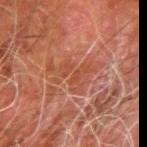Clinical impression: Captured during whole-body skin photography for melanoma surveillance; the lesion was not biopsied. Background: This is a cross-polarized tile. The lesion is on the right forearm. A male subject about 80 years old. A 15 mm close-up tile from a total-body photography series done for melanoma screening. About 6 mm across.